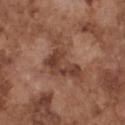size — ~7 mm (longest diameter); image source — total-body-photography crop, ~15 mm field of view; subject — male, in their mid- to late 70s; site — the chest.